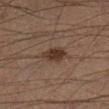biopsy status = total-body-photography surveillance lesion; no biopsy
lesion size = ≈3.5 mm
image-analysis metrics = an average lesion color of about L≈30 a*≈15 b*≈22 (CIELAB) and about 9 CIELAB-L* units darker than the surrounding skin; a nevus-likeness score of about 95/100 and a detector confidence of about 100 out of 100 that the crop contains a lesion
subject = male, aged approximately 40
lighting = cross-polarized illumination
image source = ~15 mm tile from a whole-body skin photo
site = the right lower leg